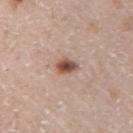Impression: No biopsy was performed on this lesion — it was imaged during a full skin examination and was not determined to be concerning. Background: The recorded lesion diameter is about 3 mm. A 15 mm close-up extracted from a 3D total-body photography capture. From the right upper arm. The patient is a female about 50 years old. The lesion-visualizer software estimated an area of roughly 4 mm², an outline eccentricity of about 0.8 (0 = round, 1 = elongated), and a shape-asymmetry score of about 0.25 (0 = symmetric). It also reported a mean CIELAB color near L≈51 a*≈20 b*≈27, roughly 16 lightness units darker than nearby skin, and a normalized lesion–skin contrast near 10.5. Imaged with white-light lighting.